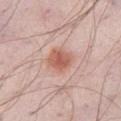Clinical impression: Imaged during a routine full-body skin examination; the lesion was not biopsied and no histopathology is available. Context: Cropped from a total-body skin-imaging series; the visible field is about 15 mm. The lesion is on the right thigh. A male patient aged approximately 55.